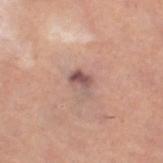A roughly 15 mm field-of-view crop from a total-body skin photograph. Located on the left leg. Captured under cross-polarized illumination. A female patient aged around 60.